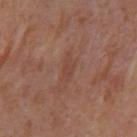Recorded during total-body skin imaging; not selected for excision or biopsy. The patient is a female aged around 50. Automated tile analysis of the lesion measured an eccentricity of roughly 0.9 and a shape-asymmetry score of about 0.25 (0 = symmetric). From the right upper arm. A 15 mm crop from a total-body photograph taken for skin-cancer surveillance. This is a cross-polarized tile. The recorded lesion diameter is about 3.5 mm.